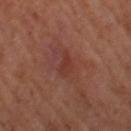Background: Located on the left thigh. Cropped from a whole-body photographic skin survey; the tile spans about 15 mm. A female patient about 65 years old. Longest diameter approximately 2.5 mm. This is a cross-polarized tile.